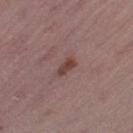Recorded during total-body skin imaging; not selected for excision or biopsy.
A female subject in their mid-40s.
A close-up tile cropped from a whole-body skin photograph, about 15 mm across.
On the left thigh.